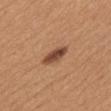{
  "site": "upper back",
  "image": {
    "source": "total-body photography crop",
    "field_of_view_mm": 15
  },
  "patient": {
    "sex": "female",
    "age_approx": 40
  },
  "lesion_size": {
    "long_diameter_mm_approx": 4.0
  }
}